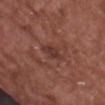biopsy_status: not biopsied; imaged during a skin examination
site: front of the torso
lighting: white-light
image:
  source: total-body photography crop
  field_of_view_mm: 15
patient:
  sex: male
  age_approx: 75
lesion_size:
  long_diameter_mm_approx: 3.0
automated_metrics:
  nevus_likeness_0_100: 0
  lesion_detection_confidence_0_100: 100